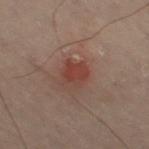Part of a total-body skin-imaging series; this lesion was reviewed on a skin check and was not flagged for biopsy. A 15 mm close-up extracted from a 3D total-body photography capture. This is a cross-polarized tile. A male subject in their 50s. On the left leg.